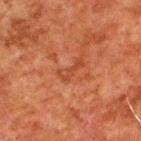Clinical impression: No biopsy was performed on this lesion — it was imaged during a full skin examination and was not determined to be concerning. Context: Imaged with cross-polarized lighting. A male patient aged 78 to 82. A 15 mm close-up tile from a total-body photography series done for melanoma screening. Located on the upper back. The lesion-visualizer software estimated a shape eccentricity near 0.9 and two-axis asymmetry of about 0.55. And it measured roughly 6 lightness units darker than nearby skin and a lesion-to-skin contrast of about 5.5 (normalized; higher = more distinct).